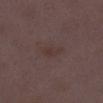| key | value |
|---|---|
| workup | catalogued during a skin exam; not biopsied |
| size | ≈3 mm |
| location | the right lower leg |
| automated metrics | an average lesion color of about L≈33 a*≈15 b*≈18 (CIELAB), a lesion–skin lightness drop of about 4, and a normalized border contrast of about 5; peripheral color asymmetry of about 0; a nevus-likeness score of about 0/100 and a detector confidence of about 100 out of 100 that the crop contains a lesion |
| patient | female, aged 28–32 |
| illumination | white-light |
| image | ~15 mm crop, total-body skin-cancer survey |